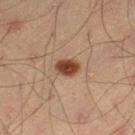biopsy status — imaged on a skin check; not biopsied
subject — male, aged 43–47
illumination — cross-polarized illumination
acquisition — ~15 mm crop, total-body skin-cancer survey
lesion diameter — ~3 mm (longest diameter)
anatomic site — the leg